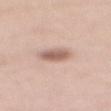Captured during whole-body skin photography for melanoma surveillance; the lesion was not biopsied.
A close-up tile cropped from a whole-body skin photograph, about 15 mm across.
From the mid back.
Measured at roughly 3 mm in maximum diameter.
The tile uses white-light illumination.
A female subject in their mid- to late 60s.